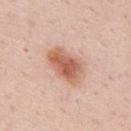Clinical impression:
Imaged during a routine full-body skin examination; the lesion was not biopsied and no histopathology is available.
Context:
This is a white-light tile. A 15 mm crop from a total-body photograph taken for skin-cancer surveillance. The subject is a male about 50 years old. About 5 mm across. On the back.